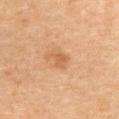A close-up tile cropped from a whole-body skin photograph, about 15 mm across.
Captured under cross-polarized illumination.
The patient is a female approximately 60 years of age.
The lesion is located on the abdomen.
Longest diameter approximately 2.5 mm.